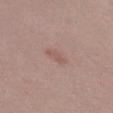A female subject, about 40 years old. The lesion's longest dimension is about 3 mm. A close-up tile cropped from a whole-body skin photograph, about 15 mm across. Located on the left thigh. The tile uses white-light illumination. An algorithmic analysis of the crop reported an area of roughly 3.5 mm², an outline eccentricity of about 0.9 (0 = round, 1 = elongated), and a symmetry-axis asymmetry near 0.25.